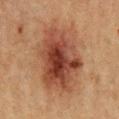Impression: Recorded during total-body skin imaging; not selected for excision or biopsy. Image and clinical context: This is a cross-polarized tile. The lesion-visualizer software estimated a shape eccentricity near 0.8 and a shape-asymmetry score of about 0.2 (0 = symmetric). It also reported a mean CIELAB color near L≈37 a*≈20 b*≈27, a lesion–skin lightness drop of about 11, and a normalized lesion–skin contrast near 9.5. It also reported a border-irregularity index near 2.5/10, a within-lesion color-variation index near 7.5/10, and a peripheral color-asymmetry measure near 2.5. And it measured an automated nevus-likeness rating near 60 out of 100 and lesion-presence confidence of about 100/100. The lesion is on the chest. A 15 mm close-up extracted from a 3D total-body photography capture. The patient is a male about 50 years old. Approximately 9.5 mm at its widest.